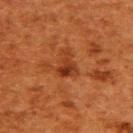Notes:
• follow-up — no biopsy performed (imaged during a skin exam)
• size — ≈3 mm
• lighting — cross-polarized
• body site — the upper back
• acquisition — total-body-photography crop, ~15 mm field of view
• patient — female, aged around 50
• automated metrics — an area of roughly 5 mm², an eccentricity of roughly 0.75, and a symmetry-axis asymmetry near 0.6; an average lesion color of about L≈31 a*≈26 b*≈33 (CIELAB), a lesion–skin lightness drop of about 8, and a normalized lesion–skin contrast near 7.5; lesion-presence confidence of about 100/100